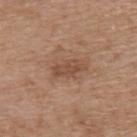This lesion was catalogued during total-body skin photography and was not selected for biopsy.
The subject is a male aged 68–72.
Approximately 4 mm at its widest.
On the upper back.
The tile uses white-light illumination.
A close-up tile cropped from a whole-body skin photograph, about 15 mm across.